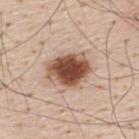The total-body-photography lesion software estimated an outline eccentricity of about 0.65 (0 = round, 1 = elongated) and a shape-asymmetry score of about 0.15 (0 = symmetric). The software also gave a mean CIELAB color near L≈51 a*≈21 b*≈29 and roughly 20 lightness units darker than nearby skin.
A male subject roughly 60 years of age.
From the upper back.
A roughly 15 mm field-of-view crop from a total-body skin photograph.
Approximately 5 mm at its widest.
The tile uses white-light illumination.